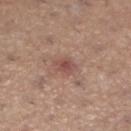{"biopsy_status": "not biopsied; imaged during a skin examination", "image": {"source": "total-body photography crop", "field_of_view_mm": 15}, "lesion_size": {"long_diameter_mm_approx": 2.5}, "automated_metrics": {"nevus_likeness_0_100": 5, "lesion_detection_confidence_0_100": 100}, "site": "left lower leg", "lighting": "white-light", "patient": {"sex": "female", "age_approx": 45}}